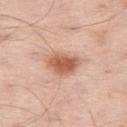The lesion was photographed on a routine skin check and not biopsied; there is no pathology result.
Located on the leg.
The recorded lesion diameter is about 4 mm.
Automated image analysis of the tile measured a lesion area of about 9 mm² and a shape-asymmetry score of about 0.25 (0 = symmetric). The analysis additionally found an average lesion color of about L≈60 a*≈23 b*≈32 (CIELAB) and a lesion-to-skin contrast of about 9 (normalized; higher = more distinct). The analysis additionally found a border-irregularity rating of about 2.5/10, a within-lesion color-variation index near 4.5/10, and a peripheral color-asymmetry measure near 1.5. The software also gave a classifier nevus-likeness of about 95/100 and lesion-presence confidence of about 100/100.
A region of skin cropped from a whole-body photographic capture, roughly 15 mm wide.
Captured under white-light illumination.
A male subject, aged around 55.